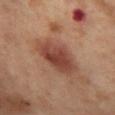Imaged during a routine full-body skin examination; the lesion was not biopsied and no histopathology is available.
The patient is a female approximately 55 years of age.
Captured under cross-polarized illumination.
Approximately 5.5 mm at its widest.
The lesion is located on the left thigh.
A roughly 15 mm field-of-view crop from a total-body skin photograph.